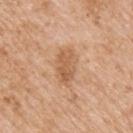| field | value |
|---|---|
| follow-up | no biopsy performed (imaged during a skin exam) |
| diameter | about 4.5 mm |
| site | the arm |
| tile lighting | white-light illumination |
| TBP lesion metrics | border irregularity of about 2.5 on a 0–10 scale, a color-variation rating of about 4/10, and peripheral color asymmetry of about 1.5; a nevus-likeness score of about 15/100 and a detector confidence of about 100 out of 100 that the crop contains a lesion |
| image | ~15 mm crop, total-body skin-cancer survey |
| subject | male, in their mid-60s |